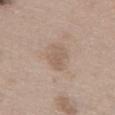From the chest. A 15 mm crop from a total-body photograph taken for skin-cancer surveillance. A female patient aged 58 to 62.